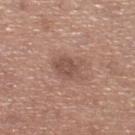workup=imaged on a skin check; not biopsied
location=the right lower leg
imaging modality=15 mm crop, total-body photography
lesion diameter=≈3.5 mm
lighting=white-light illumination
subject=female, about 55 years old